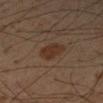<record>
  <biopsy_status>not biopsied; imaged during a skin examination</biopsy_status>
  <lighting>cross-polarized</lighting>
  <lesion_size>
    <long_diameter_mm_approx>3.0</long_diameter_mm_approx>
  </lesion_size>
  <image>
    <source>total-body photography crop</source>
    <field_of_view_mm>15</field_of_view_mm>
  </image>
  <site>right upper arm</site>
  <patient>
    <sex>male</sex>
    <age_approx>50</age_approx>
  </patient>
</record>